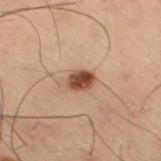follow-up: imaged on a skin check; not biopsied | diameter: about 3 mm | anatomic site: the right thigh | illumination: cross-polarized illumination | image: total-body-photography crop, ~15 mm field of view | patient: male, aged 53 to 57.